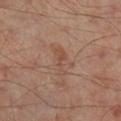Q: Was a biopsy performed?
A: no biopsy performed (imaged during a skin exam)
Q: What is the imaging modality?
A: total-body-photography crop, ~15 mm field of view
Q: Lesion location?
A: the left leg
Q: How large is the lesion?
A: about 3.5 mm
Q: Patient demographics?
A: male, aged 48–52
Q: Illumination type?
A: cross-polarized illumination